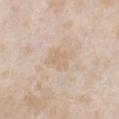Impression: No biopsy was performed on this lesion — it was imaged during a full skin examination and was not determined to be concerning. Acquisition and patient details: The patient is a female aged 23–27. A 15 mm close-up extracted from a 3D total-body photography capture. On the chest. The lesion-visualizer software estimated a lesion area of about 8 mm² and an outline eccentricity of about 0.6 (0 = round, 1 = elongated). The software also gave a mean CIELAB color near L≈68 a*≈13 b*≈30, a lesion–skin lightness drop of about 5, and a normalized lesion–skin contrast near 5. The software also gave an automated nevus-likeness rating near 0 out of 100 and a detector confidence of about 100 out of 100 that the crop contains a lesion.